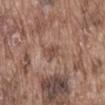Impression:
Part of a total-body skin-imaging series; this lesion was reviewed on a skin check and was not flagged for biopsy.
Clinical summary:
Approximately 3 mm at its widest. A male subject, aged 73–77. A lesion tile, about 15 mm wide, cut from a 3D total-body photograph. The tile uses white-light illumination. Located on the front of the torso.